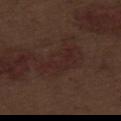Impression: This lesion was catalogued during total-body skin photography and was not selected for biopsy. Background: About 6 mm across. The lesion is on the abdomen. A male patient aged around 70. A 15 mm close-up tile from a total-body photography series done for melanoma screening.